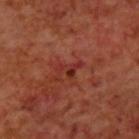A 15 mm close-up tile from a total-body photography series done for melanoma screening.
From the back.
A male patient aged approximately 70.